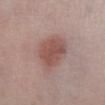Impression:
The lesion was tiled from a total-body skin photograph and was not biopsied.
Clinical summary:
The lesion is located on the right lower leg. An algorithmic analysis of the crop reported about 10 CIELAB-L* units darker than the surrounding skin. The software also gave border irregularity of about 2.5 on a 0–10 scale, a color-variation rating of about 2.5/10, and a peripheral color-asymmetry measure near 1. The patient is a female in their mid-20s. A 15 mm close-up tile from a total-body photography series done for melanoma screening.